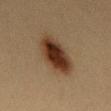Part of a total-body skin-imaging series; this lesion was reviewed on a skin check and was not flagged for biopsy. The lesion-visualizer software estimated roughly 14 lightness units darker than nearby skin and a normalized lesion–skin contrast near 13.5. The software also gave a within-lesion color-variation index near 6.5/10 and radial color variation of about 2. The lesion is on the back. The subject is a female roughly 40 years of age. A region of skin cropped from a whole-body photographic capture, roughly 15 mm wide. Longest diameter approximately 5.5 mm. Captured under cross-polarized illumination.